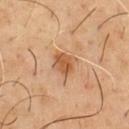This lesion was catalogued during total-body skin photography and was not selected for biopsy. On the chest. Measured at roughly 3 mm in maximum diameter. An algorithmic analysis of the crop reported a classifier nevus-likeness of about 55/100 and lesion-presence confidence of about 100/100. A 15 mm crop from a total-body photograph taken for skin-cancer surveillance. A male subject, roughly 50 years of age. Imaged with cross-polarized lighting.